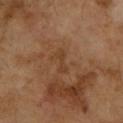Captured during whole-body skin photography for melanoma surveillance; the lesion was not biopsied. The lesion is on the left forearm. Approximately 3 mm at its widest. The patient is a female in their 70s. Cropped from a whole-body photographic skin survey; the tile spans about 15 mm.